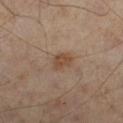Q: Was this lesion biopsied?
A: no biopsy performed (imaged during a skin exam)
Q: Illumination type?
A: cross-polarized illumination
Q: How large is the lesion?
A: about 2.5 mm
Q: How was this image acquired?
A: 15 mm crop, total-body photography
Q: What are the patient's age and sex?
A: male, in their 50s
Q: Where on the body is the lesion?
A: the left leg